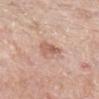Assessment:
The lesion was tiled from a total-body skin photograph and was not biopsied.
Context:
On the right lower leg. About 3 mm across. A female patient roughly 65 years of age. A region of skin cropped from a whole-body photographic capture, roughly 15 mm wide. Imaged with white-light lighting.